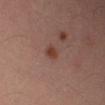Assessment: The lesion was tiled from a total-body skin photograph and was not biopsied. Acquisition and patient details: A roughly 15 mm field-of-view crop from a total-body skin photograph. The lesion is located on the right upper arm. A male subject aged around 45.